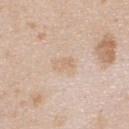– follow-up — imaged on a skin check; not biopsied
– location — the upper back
– image-analysis metrics — border irregularity of about 2.5 on a 0–10 scale, a within-lesion color-variation index near 2/10, and peripheral color asymmetry of about 0.5; lesion-presence confidence of about 100/100
– patient — male, aged around 25
– lighting — white-light
– diameter — about 2.5 mm
– image — ~15 mm tile from a whole-body skin photo